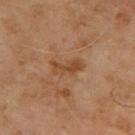This lesion was catalogued during total-body skin photography and was not selected for biopsy.
The patient is a male about 70 years old.
On the back.
Cropped from a whole-body photographic skin survey; the tile spans about 15 mm.